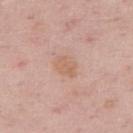Q: Was this lesion biopsied?
A: total-body-photography surveillance lesion; no biopsy
Q: Who is the patient?
A: male, aged 48 to 52
Q: How was the tile lit?
A: white-light illumination
Q: Lesion location?
A: the right upper arm
Q: Lesion size?
A: ≈3.5 mm
Q: Automated lesion metrics?
A: a footprint of about 5.5 mm² and an eccentricity of roughly 0.75; a lesion color around L≈62 a*≈20 b*≈30 in CIELAB, about 6 CIELAB-L* units darker than the surrounding skin, and a normalized lesion–skin contrast near 5.5; a border-irregularity rating of about 2.5/10, a within-lesion color-variation index near 1.5/10, and peripheral color asymmetry of about 0.5; an automated nevus-likeness rating near 5 out of 100 and a detector confidence of about 100 out of 100 that the crop contains a lesion
Q: What is the imaging modality?
A: ~15 mm tile from a whole-body skin photo